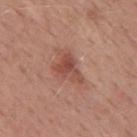Recorded during total-body skin imaging; not selected for excision or biopsy. This image is a 15 mm lesion crop taken from a total-body photograph. The patient is a male aged around 50. Imaged with white-light lighting. The lesion is on the mid back.